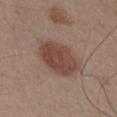<tbp_lesion>
  <biopsy_status>not biopsied; imaged during a skin examination</biopsy_status>
  <lighting>white-light</lighting>
  <patient>
    <sex>male</sex>
    <age_approx>55</age_approx>
  </patient>
  <site>chest</site>
  <image>
    <source>total-body photography crop</source>
    <field_of_view_mm>15</field_of_view_mm>
  </image>
  <automated_metrics>
    <area_mm2_approx>17.0</area_mm2_approx>
    <eccentricity>0.8</eccentricity>
    <vs_skin_darker_L>11.0</vs_skin_darker_L>
    <vs_skin_contrast_norm>8.5</vs_skin_contrast_norm>
    <border_irregularity_0_10>1.5</border_irregularity_0_10>
    <color_variation_0_10>3.0</color_variation_0_10>
    <peripheral_color_asymmetry>1.0</peripheral_color_asymmetry>
  </automated_metrics>
</tbp_lesion>